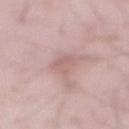biopsy status — no biopsy performed (imaged during a skin exam) | lesion diameter — ~3 mm (longest diameter) | image source — ~15 mm tile from a whole-body skin photo | TBP lesion metrics — an average lesion color of about L≈61 a*≈20 b*≈22 (CIELAB), about 7 CIELAB-L* units darker than the surrounding skin, and a lesion-to-skin contrast of about 5 (normalized; higher = more distinct); border irregularity of about 3.5 on a 0–10 scale | anatomic site — the abdomen | patient — male, aged 53 to 57 | illumination — white-light illumination.